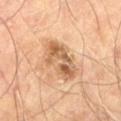Clinical impression:
No biopsy was performed on this lesion — it was imaged during a full skin examination and was not determined to be concerning.